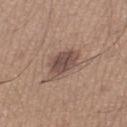  biopsy_status: not biopsied; imaged during a skin examination
  patient:
    sex: male
    age_approx: 65
  site: left thigh
  image:
    source: total-body photography crop
    field_of_view_mm: 15